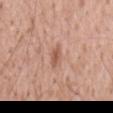{
  "biopsy_status": "not biopsied; imaged during a skin examination",
  "lighting": "white-light",
  "lesion_size": {
    "long_diameter_mm_approx": 3.0
  },
  "site": "back",
  "image": {
    "source": "total-body photography crop",
    "field_of_view_mm": 15
  },
  "patient": {
    "sex": "male",
    "age_approx": 60
  }
}